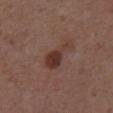<lesion>
  <biopsy_status>not biopsied; imaged during a skin examination</biopsy_status>
  <lighting>white-light</lighting>
  <lesion_size>
    <long_diameter_mm_approx>4.5</long_diameter_mm_approx>
  </lesion_size>
  <image>
    <source>total-body photography crop</source>
    <field_of_view_mm>15</field_of_view_mm>
  </image>
  <site>abdomen</site>
  <patient>
    <sex>male</sex>
    <age_approx>55</age_approx>
  </patient>
</lesion>